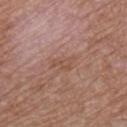<tbp_lesion>
<biopsy_status>not biopsied; imaged during a skin examination</biopsy_status>
<image>
  <source>total-body photography crop</source>
  <field_of_view_mm>15</field_of_view_mm>
</image>
<automated_metrics>
  <area_mm2_approx>4.0</area_mm2_approx>
  <eccentricity>0.8</eccentricity>
  <shape_asymmetry>0.5</shape_asymmetry>
  <cielab_L>51</cielab_L>
  <cielab_a>20</cielab_a>
  <cielab_b>28</cielab_b>
  <vs_skin_darker_L>6.0</vs_skin_darker_L>
  <vs_skin_contrast_norm>5.0</vs_skin_contrast_norm>
  <border_irregularity_0_10>5.5</border_irregularity_0_10>
  <color_variation_0_10>2.0</color_variation_0_10>
  <peripheral_color_asymmetry>1.0</peripheral_color_asymmetry>
  <nevus_likeness_0_100>0</nevus_likeness_0_100>
  <lesion_detection_confidence_0_100>100</lesion_detection_confidence_0_100>
</automated_metrics>
<site>chest</site>
<patient>
  <sex>male</sex>
  <age_approx>55</age_approx>
</patient>
<lighting>white-light</lighting>
</tbp_lesion>